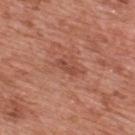notes: imaged on a skin check; not biopsied | location: the upper back | subject: male, aged around 70 | image source: 15 mm crop, total-body photography.